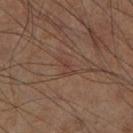biopsy status: no biopsy performed (imaged during a skin exam) | site: the right lower leg | subject: male, approximately 60 years of age | acquisition: ~15 mm crop, total-body skin-cancer survey.